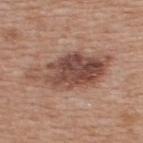Case summary:
* workup · no biopsy performed (imaged during a skin exam)
* anatomic site · the upper back
* lesion diameter · ≈9.5 mm
* image source · 15 mm crop, total-body photography
* TBP lesion metrics · a nevus-likeness score of about 75/100 and a lesion-detection confidence of about 100/100
* tile lighting · white-light illumination
* subject · male, aged 63–67